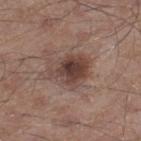biopsy status: total-body-photography surveillance lesion; no biopsy.